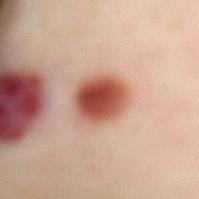Part of a total-body skin-imaging series; this lesion was reviewed on a skin check and was not flagged for biopsy. The patient is a female aged around 40. This is a cross-polarized tile. A 15 mm crop from a total-body photograph taken for skin-cancer surveillance. Automated tile analysis of the lesion measured an area of roughly 14 mm² and an eccentricity of roughly 0.55. It also reported an average lesion color of about L≈52 a*≈28 b*≈32 (CIELAB), about 18 CIELAB-L* units darker than the surrounding skin, and a lesion-to-skin contrast of about 12 (normalized; higher = more distinct). The analysis additionally found a border-irregularity rating of about 1/10, internal color variation of about 7.5 on a 0–10 scale, and a peripheral color-asymmetry measure near 2. The analysis additionally found an automated nevus-likeness rating near 100 out of 100 and lesion-presence confidence of about 100/100. From the back. Longest diameter approximately 4.5 mm.